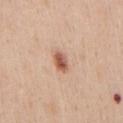Q: Is there a histopathology result?
A: total-body-photography surveillance lesion; no biopsy
Q: How large is the lesion?
A: about 2.5 mm
Q: What are the patient's age and sex?
A: male, aged around 60
Q: How was the tile lit?
A: white-light
Q: What kind of image is this?
A: ~15 mm crop, total-body skin-cancer survey
Q: What is the anatomic site?
A: the chest